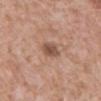| field | value |
|---|---|
| acquisition | total-body-photography crop, ~15 mm field of view |
| tile lighting | white-light illumination |
| subject | male, aged around 60 |
| location | the abdomen |